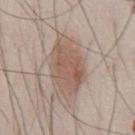follow-up: total-body-photography surveillance lesion; no biopsy | size: ≈7 mm | TBP lesion metrics: a lesion color around L≈56 a*≈16 b*≈25 in CIELAB, about 10 CIELAB-L* units darker than the surrounding skin, and a normalized border contrast of about 7; a border-irregularity index near 2.5/10; a classifier nevus-likeness of about 80/100 | location: the chest | patient: male, in their mid-40s | image: total-body-photography crop, ~15 mm field of view | tile lighting: white-light.